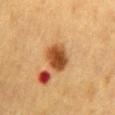Clinical impression: Captured during whole-body skin photography for melanoma surveillance; the lesion was not biopsied. Context: About 3.5 mm across. A female patient roughly 55 years of age. Located on the lower back. The tile uses cross-polarized illumination. Automated image analysis of the tile measured an average lesion color of about L≈41 a*≈21 b*≈35 (CIELAB). And it measured a classifier nevus-likeness of about 100/100 and lesion-presence confidence of about 100/100. A roughly 15 mm field-of-view crop from a total-body skin photograph.